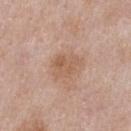workup: no biopsy performed (imaged during a skin exam)
subject: male, in their mid-50s
image: 15 mm crop, total-body photography
automated metrics: a classifier nevus-likeness of about 0/100 and lesion-presence confidence of about 100/100
location: the chest
lesion size: about 3.5 mm